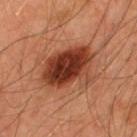Assessment:
No biopsy was performed on this lesion — it was imaged during a full skin examination and was not determined to be concerning.
Acquisition and patient details:
An algorithmic analysis of the crop reported an outline eccentricity of about 0.7 (0 = round, 1 = elongated). The software also gave a lesion color around L≈37 a*≈26 b*≈31 in CIELAB, a lesion–skin lightness drop of about 15, and a normalized lesion–skin contrast near 12. The software also gave border irregularity of about 3 on a 0–10 scale, internal color variation of about 7.5 on a 0–10 scale, and peripheral color asymmetry of about 2.5. The recorded lesion diameter is about 6.5 mm. The lesion is located on the upper back. Captured under cross-polarized illumination. A 15 mm crop from a total-body photograph taken for skin-cancer surveillance. The subject is a male roughly 45 years of age.